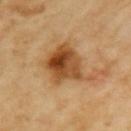Part of a total-body skin-imaging series; this lesion was reviewed on a skin check and was not flagged for biopsy. A 15 mm close-up tile from a total-body photography series done for melanoma screening. The lesion is on the left upper arm. A female patient aged 68–72.